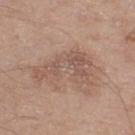Measured at roughly 6.5 mm in maximum diameter. A roughly 15 mm field-of-view crop from a total-body skin photograph. From the right thigh. A male patient aged approximately 60. Captured under white-light illumination.